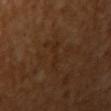From the arm.
A female patient aged around 55.
This image is a 15 mm lesion crop taken from a total-body photograph.
Measured at roughly 4 mm in maximum diameter.